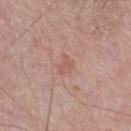| feature | finding |
|---|---|
| notes | total-body-photography surveillance lesion; no biopsy |
| acquisition | ~15 mm tile from a whole-body skin photo |
| lesion size | ≈3 mm |
| lighting | white-light |
| patient | male, about 75 years old |
| anatomic site | the right thigh |